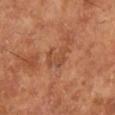On the right lower leg.
The lesion's longest dimension is about 3.5 mm.
A 15 mm crop from a total-body photograph taken for skin-cancer surveillance.
A male subject about 65 years old.
Automated tile analysis of the lesion measured a lesion area of about 6 mm² and a shape eccentricity near 0.7. It also reported a classifier nevus-likeness of about 0/100 and a detector confidence of about 100 out of 100 that the crop contains a lesion.
Imaged with cross-polarized lighting.